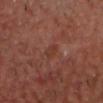Q: Was this lesion biopsied?
A: no biopsy performed (imaged during a skin exam)
Q: How was this image acquired?
A: ~15 mm crop, total-body skin-cancer survey
Q: How was the tile lit?
A: cross-polarized
Q: Lesion size?
A: ≈2.5 mm
Q: What is the anatomic site?
A: the head or neck
Q: What are the patient's age and sex?
A: male, aged 53–57